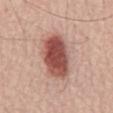{
  "biopsy_status": "not biopsied; imaged during a skin examination",
  "image": {
    "source": "total-body photography crop",
    "field_of_view_mm": 15
  },
  "site": "mid back",
  "patient": {
    "sex": "male",
    "age_approx": 70
  }
}